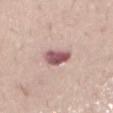{
  "lighting": "white-light",
  "site": "chest",
  "automated_metrics": {
    "border_irregularity_0_10": 2.5,
    "color_variation_0_10": 5.0,
    "peripheral_color_asymmetry": 1.5,
    "nevus_likeness_0_100": 50,
    "lesion_detection_confidence_0_100": 100
  },
  "lesion_size": {
    "long_diameter_mm_approx": 3.5
  },
  "patient": {
    "sex": "male",
    "age_approx": 60
  },
  "image": {
    "source": "total-body photography crop",
    "field_of_view_mm": 15
  }
}